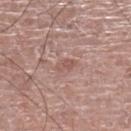Impression: Captured during whole-body skin photography for melanoma surveillance; the lesion was not biopsied. Context: The lesion-visualizer software estimated a shape eccentricity near 0.85 and a shape-asymmetry score of about 0.2 (0 = symmetric). The software also gave a classifier nevus-likeness of about 0/100. Captured under white-light illumination. A male patient, aged 73–77. The lesion is on the left lower leg. A roughly 15 mm field-of-view crop from a total-body skin photograph.